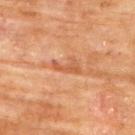workup=no biopsy performed (imaged during a skin exam) | subject=female, aged approximately 80 | imaging modality=15 mm crop, total-body photography | site=the upper back | tile lighting=cross-polarized | automated lesion analysis=a normalized border contrast of about 6; a classifier nevus-likeness of about 0/100 and a lesion-detection confidence of about 70/100.